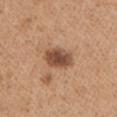Impression: Imaged during a routine full-body skin examination; the lesion was not biopsied and no histopathology is available. Clinical summary: Located on the arm. A 15 mm close-up tile from a total-body photography series done for melanoma screening. Approximately 4 mm at its widest. The subject is a male about 65 years old. The tile uses white-light illumination.